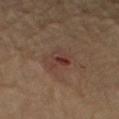Findings:
• biopsy status: catalogued during a skin exam; not biopsied
• anatomic site: the arm
• illumination: cross-polarized illumination
• image source: 15 mm crop, total-body photography
• patient: male, in their 60s